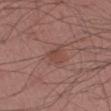No biopsy was performed on this lesion — it was imaged during a full skin examination and was not determined to be concerning. Longest diameter approximately 2.5 mm. Located on the left forearm. A male patient, about 40 years old. This is a white-light tile. This image is a 15 mm lesion crop taken from a total-body photograph.